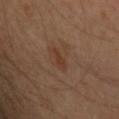biopsy status — total-body-photography surveillance lesion; no biopsy
image — ~15 mm crop, total-body skin-cancer survey
subject — male, aged approximately 45
anatomic site — the left upper arm